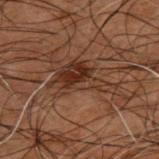Notes:
* follow-up — catalogued during a skin exam; not biopsied
* anatomic site — the upper back
* lesion diameter — ~6.5 mm (longest diameter)
* tile lighting — cross-polarized
* image source — ~15 mm crop, total-body skin-cancer survey
* subject — male, aged approximately 50